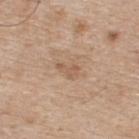Q: Lesion location?
A: the upper back
Q: How large is the lesion?
A: ~2.5 mm (longest diameter)
Q: What kind of image is this?
A: total-body-photography crop, ~15 mm field of view
Q: What lighting was used for the tile?
A: white-light
Q: Patient demographics?
A: male, aged 73–77